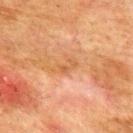Q: Was a biopsy performed?
A: no biopsy performed (imaged during a skin exam)
Q: Patient demographics?
A: male, aged 73–77
Q: What did automated image analysis measure?
A: a footprint of about 4 mm², an outline eccentricity of about 0.85 (0 = round, 1 = elongated), and a shape-asymmetry score of about 0.4 (0 = symmetric); an automated nevus-likeness rating near 0 out of 100 and a detector confidence of about 100 out of 100 that the crop contains a lesion
Q: What lighting was used for the tile?
A: cross-polarized illumination
Q: Lesion location?
A: the upper back
Q: How was this image acquired?
A: ~15 mm crop, total-body skin-cancer survey